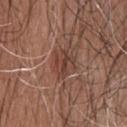workup: no biopsy performed (imaged during a skin exam) | patient: male, about 50 years old | anatomic site: the upper back | automated metrics: a lesion area of about 6.5 mm², an eccentricity of roughly 0.65, and a shape-asymmetry score of about 0.3 (0 = symmetric) | image: 15 mm crop, total-body photography.